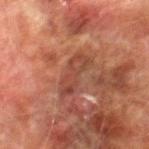A male patient, aged 73–77. The lesion's longest dimension is about 5 mm. A close-up tile cropped from a whole-body skin photograph, about 15 mm across. The lesion is located on the right thigh.